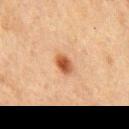notes: total-body-photography surveillance lesion; no biopsy | automated lesion analysis: an outline eccentricity of about 0.7 (0 = round, 1 = elongated) and a symmetry-axis asymmetry near 0.15; border irregularity of about 1 on a 0–10 scale, a color-variation rating of about 5/10, and peripheral color asymmetry of about 1.5; a classifier nevus-likeness of about 100/100 and a lesion-detection confidence of about 100/100 | patient: male, approximately 75 years of age | image: ~15 mm tile from a whole-body skin photo | location: the chest | size: ≈2.5 mm.